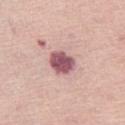notes: catalogued during a skin exam; not biopsied | location: the leg | tile lighting: white-light illumination | acquisition: ~15 mm tile from a whole-body skin photo | patient: female, aged 63 to 67 | size: about 3 mm.